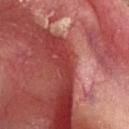A male subject, about 60 years old. The lesion is located on the head or neck. Imaged with white-light lighting. A lesion tile, about 15 mm wide, cut from a 3D total-body photograph. Measured at roughly 1.5 mm in maximum diameter. Histopathologically confirmed as a malignant lesion: squamous cell carcinoma in situ.